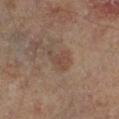Q: Was this lesion biopsied?
A: imaged on a skin check; not biopsied
Q: What is the lesion's diameter?
A: ≈3.5 mm
Q: Illumination type?
A: cross-polarized illumination
Q: Automated lesion metrics?
A: an area of roughly 5 mm², a shape eccentricity near 0.75, and a shape-asymmetry score of about 0.5 (0 = symmetric); a lesion color around L≈42 a*≈16 b*≈23 in CIELAB, roughly 6 lightness units darker than nearby skin, and a normalized border contrast of about 5; a border-irregularity rating of about 5/10 and peripheral color asymmetry of about 0.5; a detector confidence of about 100 out of 100 that the crop contains a lesion
Q: What is the anatomic site?
A: the left lower leg
Q: Who is the patient?
A: male, aged around 65
Q: How was this image acquired?
A: total-body-photography crop, ~15 mm field of view